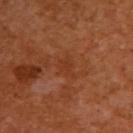biopsy status: imaged on a skin check; not biopsied
image: total-body-photography crop, ~15 mm field of view
subject: female, roughly 55 years of age
illumination: cross-polarized illumination
body site: the upper back
lesion diameter: ~2.5 mm (longest diameter)
image-analysis metrics: an area of roughly 3.5 mm² and a shape-asymmetry score of about 0.4 (0 = symmetric); a color-variation rating of about 1.5/10 and a peripheral color-asymmetry measure near 0.5; a nevus-likeness score of about 0/100 and a detector confidence of about 100 out of 100 that the crop contains a lesion